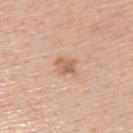Imaged during a routine full-body skin examination; the lesion was not biopsied and no histopathology is available. The patient is a male in their mid-50s. Cropped from a whole-body photographic skin survey; the tile spans about 15 mm. Imaged with white-light lighting. From the upper back.